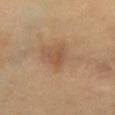workup: imaged on a skin check; not biopsied
patient: female, aged 53 to 57
illumination: cross-polarized
image: 15 mm crop, total-body photography
anatomic site: the chest